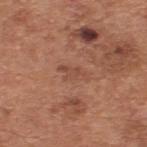The lesion was photographed on a routine skin check and not biopsied; there is no pathology result.
The subject is a male in their mid- to late 60s.
The lesion-visualizer software estimated an average lesion color of about L≈48 a*≈23 b*≈29 (CIELAB), roughly 7 lightness units darker than nearby skin, and a normalized border contrast of about 5. The analysis additionally found a nevus-likeness score of about 0/100 and a lesion-detection confidence of about 100/100.
The tile uses white-light illumination.
Cropped from a total-body skin-imaging series; the visible field is about 15 mm.
Measured at roughly 3.5 mm in maximum diameter.
From the upper back.